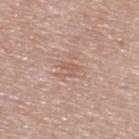image = total-body-photography crop, ~15 mm field of view
illumination = white-light
subject = male, in their mid- to late 50s
location = the upper back